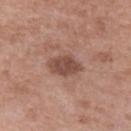{
  "biopsy_status": "not biopsied; imaged during a skin examination",
  "automated_metrics": {
    "cielab_L": 48,
    "cielab_a": 21,
    "cielab_b": 25,
    "vs_skin_darker_L": 11.0,
    "vs_skin_contrast_norm": 8.5,
    "nevus_likeness_0_100": 5
  },
  "site": "left upper arm",
  "image": {
    "source": "total-body photography crop",
    "field_of_view_mm": 15
  },
  "lesion_size": {
    "long_diameter_mm_approx": 3.5
  },
  "patient": {
    "sex": "female",
    "age_approx": 70
  }
}